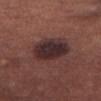The lesion was tiled from a total-body skin photograph and was not biopsied.
On the leg.
A 15 mm close-up tile from a total-body photography series done for melanoma screening.
A female patient in their mid-50s.